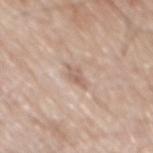Q: Was a biopsy performed?
A: imaged on a skin check; not biopsied
Q: Lesion location?
A: the mid back
Q: How was the tile lit?
A: white-light
Q: Who is the patient?
A: male, aged 78 to 82
Q: How large is the lesion?
A: ≈2.5 mm
Q: What kind of image is this?
A: total-body-photography crop, ~15 mm field of view